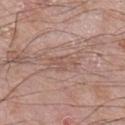| field | value |
|---|---|
| biopsy status | catalogued during a skin exam; not biopsied |
| patient | male, aged around 60 |
| body site | the right lower leg |
| imaging modality | total-body-photography crop, ~15 mm field of view |
| size | ~3.5 mm (longest diameter) |